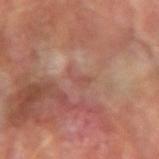{
  "biopsy_status": "not biopsied; imaged during a skin examination",
  "image": {
    "source": "total-body photography crop",
    "field_of_view_mm": 15
  },
  "site": "left forearm",
  "patient": {
    "sex": "male",
    "age_approx": 70
  },
  "automated_metrics": {
    "cielab_L": 49,
    "cielab_a": 23,
    "cielab_b": 26,
    "vs_skin_darker_L": 6.0,
    "border_irregularity_0_10": 4.5,
    "color_variation_0_10": 1.5,
    "peripheral_color_asymmetry": 0.5,
    "nevus_likeness_0_100": 0,
    "lesion_detection_confidence_0_100": 95
  }
}